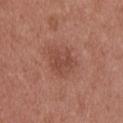Part of a total-body skin-imaging series; this lesion was reviewed on a skin check and was not flagged for biopsy.
Imaged with white-light lighting.
A lesion tile, about 15 mm wide, cut from a 3D total-body photograph.
The lesion is on the upper back.
A male patient approximately 25 years of age.